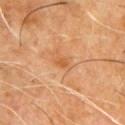  biopsy_status: not biopsied; imaged during a skin examination
  lesion_size:
    long_diameter_mm_approx: 2.0
  patient:
    sex: male
    age_approx: 60
  site: chest
  lighting: cross-polarized
  automated_metrics:
    area_mm2_approx: 1.5
    eccentricity: 0.8
    shape_asymmetry: 0.4
    cielab_L: 52
    cielab_a: 25
    cielab_b: 40
    vs_skin_darker_L: 7.0
    vs_skin_contrast_norm: 6.5
    border_irregularity_0_10: 3.5
    peripheral_color_asymmetry: 0.0
    nevus_likeness_0_100: 0
    lesion_detection_confidence_0_100: 100
  image:
    source: total-body photography crop
    field_of_view_mm: 15